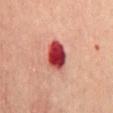Imaged during a routine full-body skin examination; the lesion was not biopsied and no histopathology is available.
The lesion is on the front of the torso.
Approximately 4 mm at its widest.
The lesion-visualizer software estimated a mean CIELAB color near L≈44 a*≈40 b*≈28 and a lesion-to-skin contrast of about 14.5 (normalized; higher = more distinct). The software also gave an automated nevus-likeness rating near 0 out of 100 and a detector confidence of about 100 out of 100 that the crop contains a lesion.
The tile uses cross-polarized illumination.
The subject is a male approximately 70 years of age.
A roughly 15 mm field-of-view crop from a total-body skin photograph.